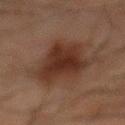workup = imaged on a skin check; not biopsied
image source = 15 mm crop, total-body photography
automated lesion analysis = a footprint of about 24 mm², an eccentricity of roughly 0.7, and a symmetry-axis asymmetry near 0.35; a mean CIELAB color near L≈25 a*≈16 b*≈21, a lesion–skin lightness drop of about 9, and a normalized lesion–skin contrast near 9.5; an automated nevus-likeness rating near 90 out of 100 and lesion-presence confidence of about 100/100
size = ≈7 mm
location = the mid back
subject = male, aged 63 to 67
illumination = cross-polarized illumination